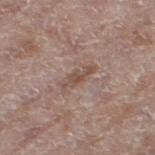Case summary:
- patient · female, approximately 75 years of age
- image-analysis metrics · an area of roughly 4.5 mm², an outline eccentricity of about 0.95 (0 = round, 1 = elongated), and a symmetry-axis asymmetry near 0.35; a border-irregularity index near 5/10 and radial color variation of about 0.5; a nevus-likeness score of about 0/100 and a lesion-detection confidence of about 95/100
- imaging modality · total-body-photography crop, ~15 mm field of view
- location · the left thigh